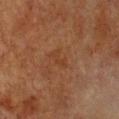Findings:
– biopsy status: imaged on a skin check; not biopsied
– site: the chest
– subject: female, aged approximately 55
– TBP lesion metrics: a footprint of about 2.5 mm² and two-axis asymmetry of about 0.45; an average lesion color of about L≈32 a*≈19 b*≈29 (CIELAB) and a normalized lesion–skin contrast near 5
– lesion diameter: about 2.5 mm
– illumination: cross-polarized illumination
– image: total-body-photography crop, ~15 mm field of view